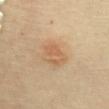biopsy status: catalogued during a skin exam; not biopsied
diameter: ~3 mm (longest diameter)
body site: the chest
illumination: cross-polarized
image source: ~15 mm crop, total-body skin-cancer survey
image-analysis metrics: an area of roughly 6 mm², an eccentricity of roughly 0.75, and two-axis asymmetry of about 0.2; a border-irregularity rating of about 2/10
patient: female, aged 63–67